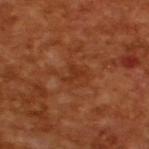Captured during whole-body skin photography for melanoma surveillance; the lesion was not biopsied. Imaged with cross-polarized lighting. Cropped from a whole-body photographic skin survey; the tile spans about 15 mm. The total-body-photography lesion software estimated an average lesion color of about L≈33 a*≈26 b*≈34 (CIELAB) and roughly 6 lightness units darker than nearby skin. The software also gave a border-irregularity rating of about 6/10, a within-lesion color-variation index near 0.5/10, and radial color variation of about 0. A male subject, aged approximately 65.